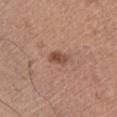{"biopsy_status": "not biopsied; imaged during a skin examination", "image": {"source": "total-body photography crop", "field_of_view_mm": 15}, "lighting": "white-light", "site": "left thigh", "patient": {"sex": "female", "age_approx": 75}, "automated_metrics": {"area_mm2_approx": 4.0, "shape_asymmetry": 0.25, "border_irregularity_0_10": 2.5, "color_variation_0_10": 3.0, "peripheral_color_asymmetry": 1.5, "nevus_likeness_0_100": 85}, "lesion_size": {"long_diameter_mm_approx": 3.0}}